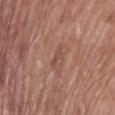<tbp_lesion>
<biopsy_status>not biopsied; imaged during a skin examination</biopsy_status>
<site>chest</site>
<automated_metrics>
  <area_mm2_approx>2.0</area_mm2_approx>
  <shape_asymmetry>0.4</shape_asymmetry>
  <border_irregularity_0_10>4.5</border_irregularity_0_10>
  <color_variation_0_10>0.0</color_variation_0_10>
</automated_metrics>
<lighting>white-light</lighting>
<image>
  <source>total-body photography crop</source>
  <field_of_view_mm>15</field_of_view_mm>
</image>
<patient>
  <sex>male</sex>
  <age_approx>65</age_approx>
</patient>
</tbp_lesion>